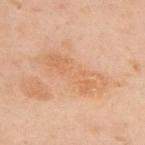biopsy status: total-body-photography surveillance lesion; no biopsy
lighting: cross-polarized
anatomic site: the upper back
patient: male, aged around 50
acquisition: ~15 mm tile from a whole-body skin photo
lesion diameter: ≈8 mm